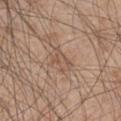Assessment:
The lesion was tiled from a total-body skin photograph and was not biopsied.